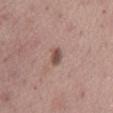{"biopsy_status": "not biopsied; imaged during a skin examination", "lesion_size": {"long_diameter_mm_approx": 2.5}, "patient": {"sex": "female", "age_approx": 65}, "site": "mid back", "image": {"source": "total-body photography crop", "field_of_view_mm": 15}, "lighting": "white-light", "automated_metrics": {"cielab_L": 50, "cielab_a": 18, "cielab_b": 23, "vs_skin_darker_L": 12.0, "vs_skin_contrast_norm": 8.5, "border_irregularity_0_10": 2.0, "color_variation_0_10": 3.0, "peripheral_color_asymmetry": 1.0}}